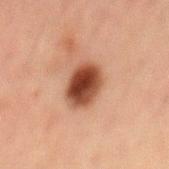Impression:
The lesion was photographed on a routine skin check and not biopsied; there is no pathology result.
Context:
The patient is a male aged 48 to 52. A close-up tile cropped from a whole-body skin photograph, about 15 mm across. Imaged with cross-polarized lighting. The lesion is on the mid back.